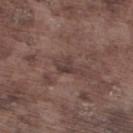Notes:
* follow-up · total-body-photography surveillance lesion; no biopsy
* body site · the leg
* imaging modality · 15 mm crop, total-body photography
* subject · male, about 75 years old